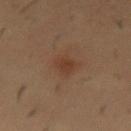Impression: Captured during whole-body skin photography for melanoma surveillance; the lesion was not biopsied.